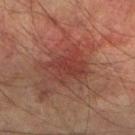<case>
<biopsy_status>not biopsied; imaged during a skin examination</biopsy_status>
<lighting>cross-polarized</lighting>
<image>
  <source>total-body photography crop</source>
  <field_of_view_mm>15</field_of_view_mm>
</image>
<patient>
  <sex>male</sex>
  <age_approx>75</age_approx>
</patient>
<site>left lower leg</site>
<automated_metrics>
  <area_mm2_approx>13.0</area_mm2_approx>
  <eccentricity>0.65</eccentricity>
  <shape_asymmetry>0.3</shape_asymmetry>
  <peripheral_color_asymmetry>1.5</peripheral_color_asymmetry>
  <nevus_likeness_0_100>15</nevus_likeness_0_100>
</automated_metrics>
</case>